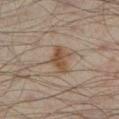biopsy_status: not biopsied; imaged during a skin examination
lighting: cross-polarized
lesion_size:
  long_diameter_mm_approx: 3.0
patient:
  sex: male
  age_approx: 45
image:
  source: total-body photography crop
  field_of_view_mm: 15
site: left lower leg